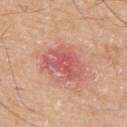Assessment: The lesion was photographed on a routine skin check and not biopsied; there is no pathology result. Background: A male subject aged 78–82. From the upper back. Automated tile analysis of the lesion measured border irregularity of about 3.5 on a 0–10 scale and a peripheral color-asymmetry measure near 1.5. Cropped from a total-body skin-imaging series; the visible field is about 15 mm.